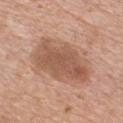Findings:
– biopsy status — total-body-photography surveillance lesion; no biopsy